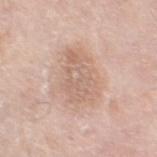Findings:
- workup — no biopsy performed (imaged during a skin exam)
- location — the left thigh
- patient — male, aged 78–82
- image source — 15 mm crop, total-body photography